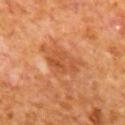Imaged during a routine full-body skin examination; the lesion was not biopsied and no histopathology is available.
A 15 mm crop from a total-body photograph taken for skin-cancer surveillance.
An algorithmic analysis of the crop reported a nevus-likeness score of about 0/100.
On the mid back.
The patient is a male aged 63 to 67.
Imaged with cross-polarized lighting.
Longest diameter approximately 4 mm.